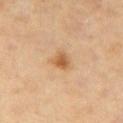Case summary:
– workup · total-body-photography surveillance lesion; no biopsy
– TBP lesion metrics · a mean CIELAB color near L≈60 a*≈22 b*≈40, a lesion–skin lightness drop of about 11, and a normalized border contrast of about 8; a classifier nevus-likeness of about 85/100 and a detector confidence of about 100 out of 100 that the crop contains a lesion
– subject · female, approximately 70 years of age
– tile lighting · cross-polarized illumination
– site · the right thigh
– image source · ~15 mm tile from a whole-body skin photo